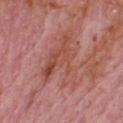workup = no biopsy performed (imaged during a skin exam) | site = the upper back | patient = male, about 65 years old | image-analysis metrics = a footprint of about 19 mm² and an eccentricity of roughly 0.7; a mean CIELAB color near L≈50 a*≈26 b*≈28, roughly 7 lightness units darker than nearby skin, and a normalized lesion–skin contrast near 6; a nevus-likeness score of about 0/100 and a detector confidence of about 65 out of 100 that the crop contains a lesion | size = ~7 mm (longest diameter) | image = ~15 mm crop, total-body skin-cancer survey | tile lighting = white-light illumination.